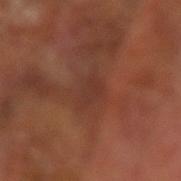Clinical impression:
Recorded during total-body skin imaging; not selected for excision or biopsy.
Image and clinical context:
From the left arm. A region of skin cropped from a whole-body photographic capture, roughly 15 mm wide. This is a cross-polarized tile. The lesion's longest dimension is about 3.5 mm. A male patient aged around 65.